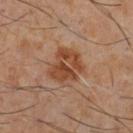Recorded during total-body skin imaging; not selected for excision or biopsy. The lesion is located on the chest. A male subject, aged around 60. A close-up tile cropped from a whole-body skin photograph, about 15 mm across.